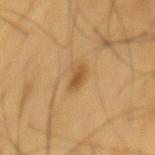Findings:
- biopsy status · catalogued during a skin exam; not biopsied
- location · the mid back
- image source · 15 mm crop, total-body photography
- lesion size · ≈3 mm
- automated metrics · a footprint of about 3.5 mm² and two-axis asymmetry of about 0.2; a mean CIELAB color near L≈53 a*≈19 b*≈41 and a lesion–skin lightness drop of about 10; a color-variation rating of about 2.5/10
- patient · male, roughly 60 years of age
- tile lighting · cross-polarized